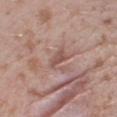Q: Patient demographics?
A: female, aged 23 to 27
Q: How was this image acquired?
A: ~15 mm crop, total-body skin-cancer survey
Q: What is the anatomic site?
A: the right thigh